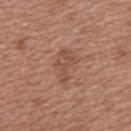notes — catalogued during a skin exam; not biopsied
site — the upper back
lighting — white-light illumination
image — total-body-photography crop, ~15 mm field of view
patient — female, in their 40s
size — ≈4 mm
automated lesion analysis — a nevus-likeness score of about 10/100